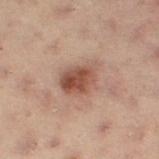Assessment: The lesion was tiled from a total-body skin photograph and was not biopsied. Image and clinical context: A female subject, aged 53 to 57. A 15 mm crop from a total-body photograph taken for skin-cancer surveillance. From the left leg.